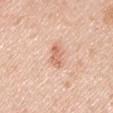Part of a total-body skin-imaging series; this lesion was reviewed on a skin check and was not flagged for biopsy. About 3 mm across. The patient is a male approximately 45 years of age. The lesion-visualizer software estimated a mean CIELAB color near L≈67 a*≈24 b*≈32, roughly 10 lightness units darker than nearby skin, and a normalized border contrast of about 6. A lesion tile, about 15 mm wide, cut from a 3D total-body photograph. The lesion is on the right upper arm.